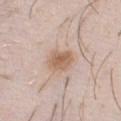Clinical impression:
This lesion was catalogued during total-body skin photography and was not selected for biopsy.
Image and clinical context:
The subject is a male aged around 30. The lesion's longest dimension is about 3 mm. The lesion is located on the chest. This is a white-light tile. A 15 mm close-up tile from a total-body photography series done for melanoma screening.